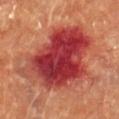Q: Was a biopsy performed?
A: no biopsy performed (imaged during a skin exam)
Q: Automated lesion metrics?
A: a nevus-likeness score of about 0/100 and lesion-presence confidence of about 100/100
Q: What is the anatomic site?
A: the chest
Q: How large is the lesion?
A: ≈9.5 mm
Q: Who is the patient?
A: female, aged approximately 80
Q: What is the imaging modality?
A: 15 mm crop, total-body photography
Q: Illumination type?
A: cross-polarized